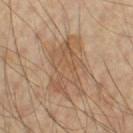{
  "image": {
    "source": "total-body photography crop",
    "field_of_view_mm": 15
  },
  "patient": {
    "sex": "male",
    "age_approx": 60
  },
  "site": "left upper arm",
  "lighting": "cross-polarized"
}